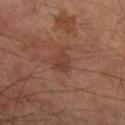notes = catalogued during a skin exam; not biopsied | location = the right lower leg | patient = male, in their 70s | lighting = cross-polarized | image = 15 mm crop, total-body photography.